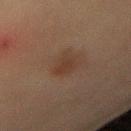automated metrics = an area of roughly 4.5 mm², a shape eccentricity near 0.7, and a symmetry-axis asymmetry near 0.25; an average lesion color of about L≈28 a*≈14 b*≈22 (CIELAB), roughly 5 lightness units darker than nearby skin, and a normalized border contrast of about 6; a color-variation rating of about 2.5/10; lesion-presence confidence of about 100/100
location = the left forearm
subject = male, approximately 65 years of age
tile lighting = cross-polarized illumination
size = ≈3 mm
acquisition = ~15 mm crop, total-body skin-cancer survey